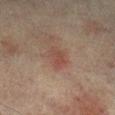<case>
<biopsy_status>not biopsied; imaged during a skin examination</biopsy_status>
<lesion_size>
  <long_diameter_mm_approx>3.0</long_diameter_mm_approx>
</lesion_size>
<site>leg</site>
<image>
  <source>total-body photography crop</source>
  <field_of_view_mm>15</field_of_view_mm>
</image>
<automated_metrics>
  <area_mm2_approx>5.0</area_mm2_approx>
  <eccentricity>0.8</eccentricity>
  <shape_asymmetry>0.3</shape_asymmetry>
  <vs_skin_darker_L>6.0</vs_skin_darker_L>
  <vs_skin_contrast_norm>5.5</vs_skin_contrast_norm>
  <border_irregularity_0_10>3.0</border_irregularity_0_10>
  <color_variation_0_10>2.5</color_variation_0_10>
  <peripheral_color_asymmetry>1.0</peripheral_color_asymmetry>
</automated_metrics>
<patient>
  <sex>male</sex>
  <age_approx>75</age_approx>
</patient>
<lighting>cross-polarized</lighting>
</case>